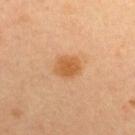  biopsy_status: not biopsied; imaged during a skin examination
  lighting: cross-polarized
  image:
    source: total-body photography crop
    field_of_view_mm: 15
  lesion_size:
    long_diameter_mm_approx: 3.0
  site: upper back
  automated_metrics:
    area_mm2_approx: 6.5
    eccentricity: 0.6
    border_irregularity_0_10: 2.0
    nevus_likeness_0_100: 95
    lesion_detection_confidence_0_100: 100
  patient:
    sex: female
    age_approx: 35